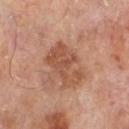Q: Is there a histopathology result?
A: imaged on a skin check; not biopsied
Q: Who is the patient?
A: male, aged 63–67
Q: What lighting was used for the tile?
A: cross-polarized
Q: Automated lesion metrics?
A: a lesion area of about 13 mm², an outline eccentricity of about 0.8 (0 = round, 1 = elongated), and a symmetry-axis asymmetry near 0.35; a color-variation rating of about 3/10 and radial color variation of about 1
Q: What is the imaging modality?
A: ~15 mm crop, total-body skin-cancer survey
Q: Where on the body is the lesion?
A: the left lower leg
Q: How large is the lesion?
A: ~5 mm (longest diameter)